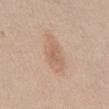biopsy status: no biopsy performed (imaged during a skin exam) | diameter: about 5 mm | automated metrics: an area of roughly 10 mm²; an average lesion color of about L≈62 a*≈18 b*≈30 (CIELAB), a lesion–skin lightness drop of about 8, and a normalized border contrast of about 5.5; a border-irregularity index near 2.5/10 and a color-variation rating of about 2.5/10; a classifier nevus-likeness of about 50/100 and lesion-presence confidence of about 100/100 | tile lighting: white-light | body site: the abdomen | image source: total-body-photography crop, ~15 mm field of view | subject: female, aged approximately 45.